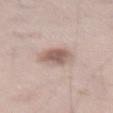Impression: The lesion was photographed on a routine skin check and not biopsied; there is no pathology result. Image and clinical context: Captured under white-light illumination. On the left thigh. A male patient, about 55 years old. Cropped from a whole-body photographic skin survey; the tile spans about 15 mm.